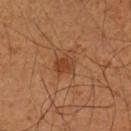{"biopsy_status": "not biopsied; imaged during a skin examination", "lighting": "cross-polarized", "automated_metrics": {"area_mm2_approx": 5.0, "eccentricity": 0.55, "shape_asymmetry": 0.2, "cielab_L": 39, "cielab_a": 23, "cielab_b": 33, "vs_skin_darker_L": 8.0, "vs_skin_contrast_norm": 6.5, "border_irregularity_0_10": 1.5, "color_variation_0_10": 2.5, "peripheral_color_asymmetry": 1.0, "nevus_likeness_0_100": 65}, "image": {"source": "total-body photography crop", "field_of_view_mm": 15}, "site": "arm", "lesion_size": {"long_diameter_mm_approx": 2.5}, "patient": {"sex": "male", "age_approx": 50}}